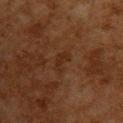Impression: Imaged during a routine full-body skin examination; the lesion was not biopsied and no histopathology is available. Background: The lesion is located on the upper back. A region of skin cropped from a whole-body photographic capture, roughly 15 mm wide. The tile uses cross-polarized illumination. A male subject, roughly 65 years of age.